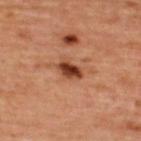The lesion was photographed on a routine skin check and not biopsied; there is no pathology result.
A 15 mm crop from a total-body photograph taken for skin-cancer surveillance.
The lesion is located on the back.
Measured at roughly 3 mm in maximum diameter.
The patient is a female aged approximately 45.